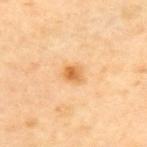Clinical summary:
The lesion is on the upper back. This image is a 15 mm lesion crop taken from a total-body photograph. Measured at roughly 2.5 mm in maximum diameter. The patient is a male roughly 65 years of age.